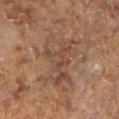{"biopsy_status": "not biopsied; imaged during a skin examination", "patient": {"sex": "female"}, "lesion_size": {"long_diameter_mm_approx": 6.0}, "site": "left lower leg", "image": {"source": "total-body photography crop", "field_of_view_mm": 15}}